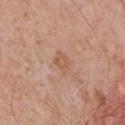follow-up: catalogued during a skin exam; not biopsied | subject: male, aged approximately 60 | anatomic site: the chest | image source: ~15 mm crop, total-body skin-cancer survey.